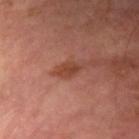No biopsy was performed on this lesion — it was imaged during a full skin examination and was not determined to be concerning. On the left forearm. About 2.5 mm across. A male patient, aged 63–67. This is a cross-polarized tile. A 15 mm close-up tile from a total-body photography series done for melanoma screening.